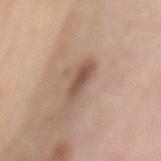{"automated_metrics": {"area_mm2_approx": 6.0, "eccentricity": 0.9, "shape_asymmetry": 0.2, "nevus_likeness_0_100": 45}, "site": "back", "image": {"source": "total-body photography crop", "field_of_view_mm": 15}, "lighting": "white-light", "patient": {"sex": "female", "age_approx": 70}, "lesion_size": {"long_diameter_mm_approx": 4.0}}